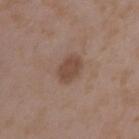Impression:
Part of a total-body skin-imaging series; this lesion was reviewed on a skin check and was not flagged for biopsy.
Background:
Cropped from a total-body skin-imaging series; the visible field is about 15 mm. On the left upper arm. This is a white-light tile. The recorded lesion diameter is about 3.5 mm. A female subject, aged around 35.